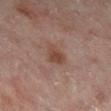biopsy status = no biopsy performed (imaged during a skin exam) | image = total-body-photography crop, ~15 mm field of view | diameter = ~3 mm (longest diameter) | tile lighting = cross-polarized | site = the right lower leg | patient = male, approximately 65 years of age.